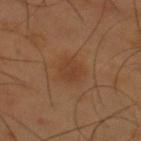The lesion was photographed on a routine skin check and not biopsied; there is no pathology result. The lesion is on the upper back. An algorithmic analysis of the crop reported internal color variation of about 2.5 on a 0–10 scale and radial color variation of about 1. Imaged with cross-polarized lighting. A male patient aged approximately 55. The lesion's longest dimension is about 3 mm. This image is a 15 mm lesion crop taken from a total-body photograph.